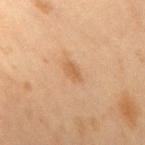biopsy_status: not biopsied; imaged during a skin examination
patient:
  sex: female
  age_approx: 45
lighting: cross-polarized
image:
  source: total-body photography crop
  field_of_view_mm: 15
site: right upper arm